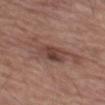<record>
<biopsy_status>not biopsied; imaged during a skin examination</biopsy_status>
<site>leg</site>
<lesion_size>
  <long_diameter_mm_approx>7.5</long_diameter_mm_approx>
</lesion_size>
<patient>
  <sex>male</sex>
  <age_approx>65</age_approx>
</patient>
<automated_metrics>
  <cielab_L>44</cielab_L>
  <cielab_a>19</cielab_a>
  <cielab_b>23</cielab_b>
  <vs_skin_darker_L>9.0</vs_skin_darker_L>
  <vs_skin_contrast_norm>7.5</vs_skin_contrast_norm>
  <lesion_detection_confidence_0_100>100</lesion_detection_confidence_0_100>
</automated_metrics>
<image>
  <source>total-body photography crop</source>
  <field_of_view_mm>15</field_of_view_mm>
</image>
</record>